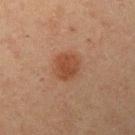The lesion was tiled from a total-body skin photograph and was not biopsied.
A close-up tile cropped from a whole-body skin photograph, about 15 mm across.
A male subject, approximately 60 years of age.
Measured at roughly 3 mm in maximum diameter.
The lesion is located on the left upper arm.